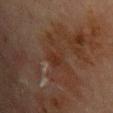Impression: Captured during whole-body skin photography for melanoma surveillance; the lesion was not biopsied. Acquisition and patient details: About 5 mm across. A male patient aged around 70. The lesion is on the back. A 15 mm close-up extracted from a 3D total-body photography capture.